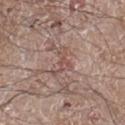{
  "biopsy_status": "not biopsied; imaged during a skin examination",
  "image": {
    "source": "total-body photography crop",
    "field_of_view_mm": 15
  },
  "lighting": "white-light",
  "lesion_size": {
    "long_diameter_mm_approx": 4.0
  },
  "site": "leg",
  "patient": {
    "sex": "male",
    "age_approx": 60
  }
}